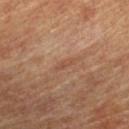notes: imaged on a skin check; not biopsied | image: total-body-photography crop, ~15 mm field of view | size: about 2.5 mm | tile lighting: cross-polarized | location: the right lower leg | patient: male, aged approximately 85 or older.